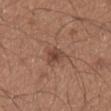notes: imaged on a skin check; not biopsied
patient: male, in their mid- to late 20s
tile lighting: white-light
body site: the chest
size: ~3 mm (longest diameter)
acquisition: 15 mm crop, total-body photography